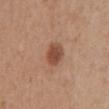Clinical impression:
Captured during whole-body skin photography for melanoma surveillance; the lesion was not biopsied.
Clinical summary:
The recorded lesion diameter is about 3.5 mm. The lesion is on the mid back. A female subject roughly 40 years of age. Cropped from a whole-body photographic skin survey; the tile spans about 15 mm. Imaged with white-light lighting.